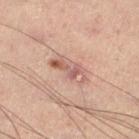<tbp_lesion>
<biopsy_status>not biopsied; imaged during a skin examination</biopsy_status>
<site>left lower leg</site>
<patient>
  <sex>male</sex>
  <age_approx>50</age_approx>
</patient>
<lighting>cross-polarized</lighting>
<lesion_size>
  <long_diameter_mm_approx>4.5</long_diameter_mm_approx>
</lesion_size>
<image>
  <source>total-body photography crop</source>
  <field_of_view_mm>15</field_of_view_mm>
</image>
</tbp_lesion>